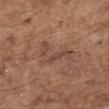Impression: The lesion was tiled from a total-body skin photograph and was not biopsied. Clinical summary: A region of skin cropped from a whole-body photographic capture, roughly 15 mm wide. From the mid back. Captured under white-light illumination. A female patient approximately 65 years of age. The lesion's longest dimension is about 5.5 mm.